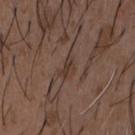The lesion was tiled from a total-body skin photograph and was not biopsied. Captured under white-light illumination. A 15 mm close-up tile from a total-body photography series done for melanoma screening. A male patient in their 50s. Longest diameter approximately 2.5 mm. The lesion is on the front of the torso. An algorithmic analysis of the crop reported a footprint of about 3 mm² and an outline eccentricity of about 0.85 (0 = round, 1 = elongated). It also reported a border-irregularity rating of about 4.5/10, a color-variation rating of about 0/10, and peripheral color asymmetry of about 0.